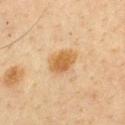workup: catalogued during a skin exam; not biopsied | automated lesion analysis: a mean CIELAB color near L≈55 a*≈18 b*≈39 and roughly 10 lightness units darker than nearby skin; lesion-presence confidence of about 100/100 | size: about 3.5 mm | acquisition: 15 mm crop, total-body photography | illumination: cross-polarized illumination | subject: male, in their 60s | body site: the front of the torso.